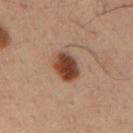lesion size = ~4 mm (longest diameter)
lighting = cross-polarized illumination
image source = ~15 mm tile from a whole-body skin photo
patient = male, aged 33–37
body site = the right upper arm